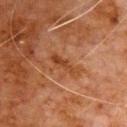No biopsy was performed on this lesion — it was imaged during a full skin examination and was not determined to be concerning. The lesion-visualizer software estimated a lesion color around L≈34 a*≈22 b*≈30 in CIELAB, a lesion–skin lightness drop of about 7, and a lesion-to-skin contrast of about 7 (normalized; higher = more distinct). The software also gave a color-variation rating of about 0.5/10. Longest diameter approximately 4 mm. A male subject, aged around 80. Cropped from a total-body skin-imaging series; the visible field is about 15 mm. This is a cross-polarized tile. The lesion is on the chest.